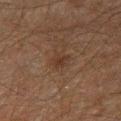Impression: The lesion was tiled from a total-body skin photograph and was not biopsied. Image and clinical context: From the leg. The patient is a male approximately 60 years of age. About 2.5 mm across. This is a cross-polarized tile. A lesion tile, about 15 mm wide, cut from a 3D total-body photograph.